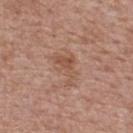| field | value |
|---|---|
| biopsy status | imaged on a skin check; not biopsied |
| site | the upper back |
| automated lesion analysis | a mean CIELAB color near L≈53 a*≈20 b*≈29, a lesion–skin lightness drop of about 7, and a lesion-to-skin contrast of about 5.5 (normalized; higher = more distinct); a border-irregularity index near 6/10, a within-lesion color-variation index near 3.5/10, and radial color variation of about 1; a nevus-likeness score of about 0/100 and lesion-presence confidence of about 100/100 |
| image source | total-body-photography crop, ~15 mm field of view |
| patient | female, in their mid-60s |
| diameter | ~4.5 mm (longest diameter) |
| tile lighting | white-light illumination |